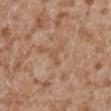<record>
  <biopsy_status>not biopsied; imaged during a skin examination</biopsy_status>
  <image>
    <source>total-body photography crop</source>
    <field_of_view_mm>15</field_of_view_mm>
  </image>
  <lesion_size>
    <long_diameter_mm_approx>2.5</long_diameter_mm_approx>
  </lesion_size>
  <patient>
    <sex>male</sex>
    <age_approx>45</age_approx>
  </patient>
  <automated_metrics>
    <nevus_likeness_0_100>0</nevus_likeness_0_100>
    <lesion_detection_confidence_0_100>90</lesion_detection_confidence_0_100>
  </automated_metrics>
  <lighting>white-light</lighting>
  <site>abdomen</site>
</record>